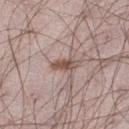Recorded during total-body skin imaging; not selected for excision or biopsy. From the leg. An algorithmic analysis of the crop reported a footprint of about 3.5 mm². The software also gave a border-irregularity index near 3.5/10 and peripheral color asymmetry of about 0.5. Cropped from a total-body skin-imaging series; the visible field is about 15 mm. A male patient aged around 25.